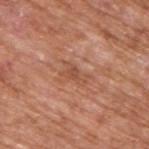This lesion was catalogued during total-body skin photography and was not selected for biopsy. A roughly 15 mm field-of-view crop from a total-body skin photograph. A male patient aged 63–67. Automated image analysis of the tile measured a border-irregularity rating of about 4.5/10, a within-lesion color-variation index near 2/10, and radial color variation of about 0.5. Approximately 3 mm at its widest. Imaged with white-light lighting. On the upper back.